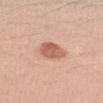{"biopsy_status": "not biopsied; imaged during a skin examination", "image": {"source": "total-body photography crop", "field_of_view_mm": 15}, "site": "left forearm", "patient": {"sex": "female", "age_approx": 40}}